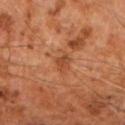No biopsy was performed on this lesion — it was imaged during a full skin examination and was not determined to be concerning.
A region of skin cropped from a whole-body photographic capture, roughly 15 mm wide.
The lesion is located on the arm.
The tile uses cross-polarized illumination.
Measured at roughly 2.5 mm in maximum diameter.
Automated image analysis of the tile measured an area of roughly 3 mm², an outline eccentricity of about 0.8 (0 = round, 1 = elongated), and two-axis asymmetry of about 0.35. It also reported a border-irregularity index near 3.5/10, a within-lesion color-variation index near 1/10, and a peripheral color-asymmetry measure near 0.5. The analysis additionally found an automated nevus-likeness rating near 0 out of 100 and a lesion-detection confidence of about 100/100.
A male patient, roughly 60 years of age.